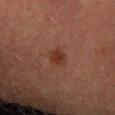The lesion was photographed on a routine skin check and not biopsied; there is no pathology result. The lesion-visualizer software estimated an average lesion color of about L≈27 a*≈19 b*≈24 (CIELAB) and a normalized lesion–skin contrast near 7.5. And it measured a classifier nevus-likeness of about 90/100. Cropped from a total-body skin-imaging series; the visible field is about 15 mm. On the right forearm. About 2.5 mm across. Imaged with cross-polarized lighting. A female subject, roughly 55 years of age.